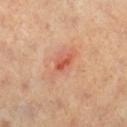Imaged during a routine full-body skin examination; the lesion was not biopsied and no histopathology is available.
A female subject, approximately 40 years of age.
Cropped from a whole-body photographic skin survey; the tile spans about 15 mm.
About 2.5 mm across.
Captured under cross-polarized illumination.
From the right lower leg.
An algorithmic analysis of the crop reported an outline eccentricity of about 0.9 (0 = round, 1 = elongated) and a symmetry-axis asymmetry near 0.3.